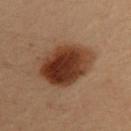<case>
  <biopsy_status>not biopsied; imaged during a skin examination</biopsy_status>
  <lighting>cross-polarized</lighting>
  <image>
    <source>total-body photography crop</source>
    <field_of_view_mm>15</field_of_view_mm>
  </image>
  <automated_metrics>
    <border_irregularity_0_10>1.5</border_irregularity_0_10>
    <color_variation_0_10>8.0</color_variation_0_10>
    <peripheral_color_asymmetry>2.5</peripheral_color_asymmetry>
  </automated_metrics>
  <site>upper back</site>
  <lesion_size>
    <long_diameter_mm_approx>6.5</long_diameter_mm_approx>
  </lesion_size>
  <patient>
    <sex>female</sex>
    <age_approx>35</age_approx>
  </patient>
</case>